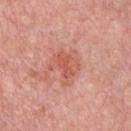workup: imaged on a skin check; not biopsied
image source: total-body-photography crop, ~15 mm field of view
site: the chest
patient: male, approximately 45 years of age
lesion diameter: ≈3.5 mm
tile lighting: white-light illumination